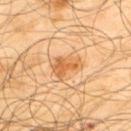Case summary:
• workup — imaged on a skin check; not biopsied
• subject — male, in their mid-60s
• size — ~3.5 mm (longest diameter)
• location — the upper back
• image-analysis metrics — a lesion area of about 6.5 mm², a shape eccentricity near 0.7, and two-axis asymmetry of about 0.45
• image source — 15 mm crop, total-body photography
• tile lighting — cross-polarized